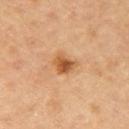{
  "biopsy_status": "not biopsied; imaged during a skin examination",
  "patient": {
    "sex": "male",
    "age_approx": "85+"
  },
  "site": "right upper arm",
  "image": {
    "source": "total-body photography crop",
    "field_of_view_mm": 15
  },
  "lighting": "cross-polarized",
  "lesion_size": {
    "long_diameter_mm_approx": 2.5
  }
}